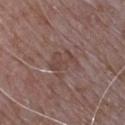Recorded during total-body skin imaging; not selected for excision or biopsy. A male patient aged around 65. A close-up tile cropped from a whole-body skin photograph, about 15 mm across. Located on the chest.